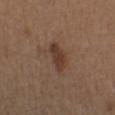{
  "biopsy_status": "not biopsied; imaged during a skin examination"
}